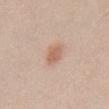subject = male, aged around 25 | site = the front of the torso | acquisition = total-body-photography crop, ~15 mm field of view.